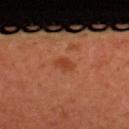The patient is a female aged around 40. Cropped from a total-body skin-imaging series; the visible field is about 15 mm. The total-body-photography lesion software estimated an area of roughly 3.5 mm², an eccentricity of roughly 0.8, and two-axis asymmetry of about 0.2. The analysis additionally found an average lesion color of about L≈43 a*≈29 b*≈37 (CIELAB), roughly 7 lightness units darker than nearby skin, and a normalized border contrast of about 6. It also reported peripheral color asymmetry of about 0.5. The lesion is on the back. The tile uses cross-polarized illumination.